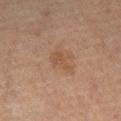patient: male, aged approximately 50
diameter: about 2.5 mm
automated lesion analysis: an area of roughly 3.5 mm² and a shape-asymmetry score of about 0.45 (0 = symmetric); a lesion–skin lightness drop of about 6 and a normalized border contrast of about 5.5
acquisition: ~15 mm tile from a whole-body skin photo
body site: the left leg
lighting: cross-polarized illumination